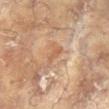<tbp_lesion>
<biopsy_status>not biopsied; imaged during a skin examination</biopsy_status>
<site>right arm</site>
<image>
  <source>total-body photography crop</source>
  <field_of_view_mm>15</field_of_view_mm>
</image>
<patient>
  <sex>female</sex>
  <age_approx>80</age_approx>
</patient>
</tbp_lesion>